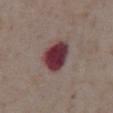Assessment:
The lesion was photographed on a routine skin check and not biopsied; there is no pathology result.
Context:
About 4 mm across. The patient is a male aged 73–77. The total-body-photography lesion software estimated an area of roughly 11 mm², an eccentricity of roughly 0.65, and a symmetry-axis asymmetry near 0.2. The analysis additionally found an average lesion color of about L≈35 a*≈26 b*≈14 (CIELAB), roughly 19 lightness units darker than nearby skin, and a lesion-to-skin contrast of about 15.5 (normalized; higher = more distinct). The analysis additionally found a color-variation rating of about 5/10 and peripheral color asymmetry of about 1.5. Imaged with white-light lighting. On the front of the torso. A 15 mm crop from a total-body photograph taken for skin-cancer surveillance.